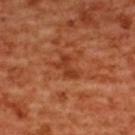biopsy_status: not biopsied; imaged during a skin examination
patient:
  sex: female
  age_approx: 55
lesion_size:
  long_diameter_mm_approx: 3.0
lighting: cross-polarized
site: upper back
image:
  source: total-body photography crop
  field_of_view_mm: 15